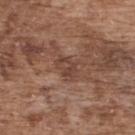notes = catalogued during a skin exam; not biopsied
anatomic site = the upper back
subject = male, roughly 75 years of age
acquisition = ~15 mm tile from a whole-body skin photo
illumination = white-light illumination